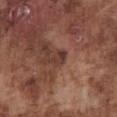Assessment: The lesion was tiled from a total-body skin photograph and was not biopsied. Context: A 15 mm close-up extracted from a 3D total-body photography capture. A male patient aged 73 to 77. Automated image analysis of the tile measured a footprint of about 2.5 mm² and a shape eccentricity near 0.85. The software also gave about 9 CIELAB-L* units darker than the surrounding skin. The analysis additionally found border irregularity of about 4 on a 0–10 scale and a within-lesion color-variation index near 0/10. The software also gave lesion-presence confidence of about 90/100. Imaged with white-light lighting. On the abdomen. Approximately 2.5 mm at its widest.